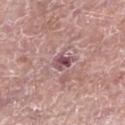Recorded during total-body skin imaging; not selected for excision or biopsy. An algorithmic analysis of the crop reported roughly 12 lightness units darker than nearby skin and a normalized lesion–skin contrast near 9. The analysis additionally found an automated nevus-likeness rating near 5 out of 100 and a lesion-detection confidence of about 100/100. Measured at roughly 2.5 mm in maximum diameter. The lesion is located on the leg. A lesion tile, about 15 mm wide, cut from a 3D total-body photograph. A male patient in their mid- to late 60s.